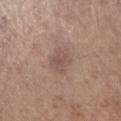The lesion was tiled from a total-body skin photograph and was not biopsied.